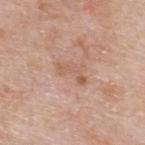{"biopsy_status": "not biopsied; imaged during a skin examination", "site": "upper back", "image": {"source": "total-body photography crop", "field_of_view_mm": 15}, "lesion_size": {"long_diameter_mm_approx": 3.5}, "automated_metrics": {"area_mm2_approx": 4.5, "eccentricity": 0.85, "vs_skin_darker_L": 7.0, "vs_skin_contrast_norm": 5.5, "border_irregularity_0_10": 8.0, "color_variation_0_10": 0.0, "peripheral_color_asymmetry": 0.0, "nevus_likeness_0_100": 0, "lesion_detection_confidence_0_100": 100}, "patient": {"sex": "male", "age_approx": 60}, "lighting": "white-light"}